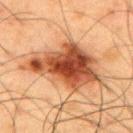The lesion was tiled from a total-body skin photograph and was not biopsied. An algorithmic analysis of the crop reported an area of roughly 29 mm² and two-axis asymmetry of about 0.4. And it measured a border-irregularity index near 5/10, a within-lesion color-variation index near 7.5/10, and a peripheral color-asymmetry measure near 2.5. The software also gave a nevus-likeness score of about 90/100. A roughly 15 mm field-of-view crop from a total-body skin photograph. A male subject aged 58 to 62. The lesion is located on the upper back. The tile uses cross-polarized illumination.